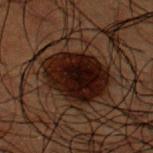biopsy status = no biopsy performed (imaged during a skin exam)
body site = the upper back
subject = male, aged around 50
image = ~15 mm tile from a whole-body skin photo